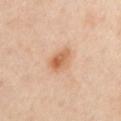Q: Is there a histopathology result?
A: catalogued during a skin exam; not biopsied
Q: What is the lesion's diameter?
A: about 3.5 mm
Q: What kind of image is this?
A: total-body-photography crop, ~15 mm field of view
Q: Patient demographics?
A: male, about 65 years old
Q: What lighting was used for the tile?
A: cross-polarized illumination
Q: Where on the body is the lesion?
A: the chest